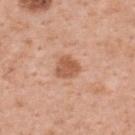Clinical impression: Captured during whole-body skin photography for melanoma surveillance; the lesion was not biopsied. Acquisition and patient details: Longest diameter approximately 3 mm. The patient is a female roughly 50 years of age. A roughly 15 mm field-of-view crop from a total-body skin photograph. The lesion is on the upper back. Imaged with white-light lighting. Automated tile analysis of the lesion measured an average lesion color of about L≈57 a*≈24 b*≈33 (CIELAB), about 11 CIELAB-L* units darker than the surrounding skin, and a normalized lesion–skin contrast near 7.5. The analysis additionally found border irregularity of about 2.5 on a 0–10 scale and peripheral color asymmetry of about 0.5. The analysis additionally found an automated nevus-likeness rating near 25 out of 100 and a detector confidence of about 100 out of 100 that the crop contains a lesion.